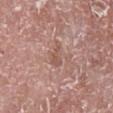workup: no biopsy performed (imaged during a skin exam)
TBP lesion metrics: a footprint of about 4 mm², a shape eccentricity near 0.75, and a symmetry-axis asymmetry near 0.45; a mean CIELAB color near L≈54 a*≈21 b*≈25, a lesion–skin lightness drop of about 7, and a lesion-to-skin contrast of about 5 (normalized; higher = more distinct); a border-irregularity rating of about 4.5/10, a color-variation rating of about 1.5/10, and peripheral color asymmetry of about 0.5; a classifier nevus-likeness of about 0/100 and lesion-presence confidence of about 100/100
patient: male, roughly 75 years of age
anatomic site: the leg
acquisition: ~15 mm tile from a whole-body skin photo
lighting: white-light illumination